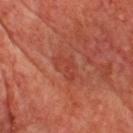follow-up = imaged on a skin check; not biopsied
subject = male, about 65 years old
automated metrics = a lesion area of about 4.5 mm²; a lesion color around L≈42 a*≈32 b*≈32 in CIELAB, roughly 6 lightness units darker than nearby skin, and a normalized border contrast of about 5; an automated nevus-likeness rating near 0 out of 100 and lesion-presence confidence of about 100/100
image = 15 mm crop, total-body photography
lighting = cross-polarized illumination
anatomic site = the chest
lesion diameter = ~3.5 mm (longest diameter)